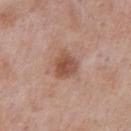This lesion was catalogued during total-body skin photography and was not selected for biopsy.
A region of skin cropped from a whole-body photographic capture, roughly 15 mm wide.
This is a white-light tile.
A male patient, in their mid-50s.
The lesion is on the right upper arm.
The recorded lesion diameter is about 3.5 mm.